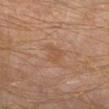Imaged during a routine full-body skin examination; the lesion was not biopsied and no histopathology is available. Longest diameter approximately 3 mm. A close-up tile cropped from a whole-body skin photograph, about 15 mm across. Captured under cross-polarized illumination. From the right lower leg. A male subject, aged 58–62.